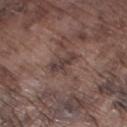Part of a total-body skin-imaging series; this lesion was reviewed on a skin check and was not flagged for biopsy. The lesion is on the left lower leg. A male patient, aged approximately 75. Longest diameter approximately 3.5 mm. A 15 mm crop from a total-body photograph taken for skin-cancer surveillance. Automated image analysis of the tile measured a lesion area of about 5 mm², an eccentricity of roughly 0.85, and a symmetry-axis asymmetry near 0.45. The analysis additionally found a mean CIELAB color near L≈38 a*≈16 b*≈18, a lesion–skin lightness drop of about 9, and a normalized border contrast of about 8. And it measured a nevus-likeness score of about 0/100.